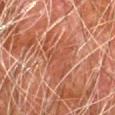The lesion was tiled from a total-body skin photograph and was not biopsied.
A male subject aged around 80.
From the left forearm.
A close-up tile cropped from a whole-body skin photograph, about 15 mm across.
Automated image analysis of the tile measured an average lesion color of about L≈40 a*≈23 b*≈28 (CIELAB) and a normalized border contrast of about 5. The software also gave a border-irregularity rating of about 7/10, a within-lesion color-variation index near 2.5/10, and radial color variation of about 1.
Imaged with cross-polarized lighting.